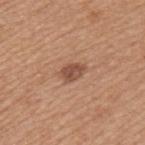<lesion>
  <biopsy_status>not biopsied; imaged during a skin examination</biopsy_status>
  <image>
    <source>total-body photography crop</source>
    <field_of_view_mm>15</field_of_view_mm>
  </image>
  <site>left upper arm</site>
  <patient>
    <sex>male</sex>
    <age_approx>65</age_approx>
  </patient>
</lesion>